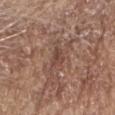Impression: Imaged during a routine full-body skin examination; the lesion was not biopsied and no histopathology is available. Clinical summary: Longest diameter approximately 2.5 mm. From the head or neck. Imaged with white-light lighting. Automated tile analysis of the lesion measured a border-irregularity rating of about 3.5/10 and a color-variation rating of about 2/10. The subject is a male in their mid- to late 60s. A region of skin cropped from a whole-body photographic capture, roughly 15 mm wide.